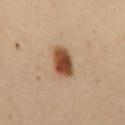A female patient roughly 50 years of age. The lesion is on the abdomen. Cropped from a total-body skin-imaging series; the visible field is about 15 mm. Imaged with cross-polarized lighting. Longest diameter approximately 4.5 mm. The lesion-visualizer software estimated an area of roughly 9 mm², a shape eccentricity near 0.8, and two-axis asymmetry of about 0.15. And it measured border irregularity of about 1.5 on a 0–10 scale. The analysis additionally found an automated nevus-likeness rating near 100 out of 100 and lesion-presence confidence of about 100/100.